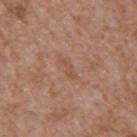| key | value |
|---|---|
| follow-up | no biopsy performed (imaged during a skin exam) |
| lighting | white-light illumination |
| image source | ~15 mm crop, total-body skin-cancer survey |
| anatomic site | the mid back |
| patient | male, aged around 65 |
| automated metrics | a footprint of about 2.5 mm², a shape eccentricity near 0.95, and two-axis asymmetry of about 0.5; an average lesion color of about L≈51 a*≈22 b*≈30 (CIELAB), a lesion–skin lightness drop of about 6, and a normalized border contrast of about 4.5; an automated nevus-likeness rating near 0 out of 100 and a lesion-detection confidence of about 100/100 |
| lesion diameter | ~3 mm (longest diameter) |